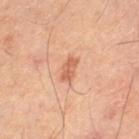Part of a total-body skin-imaging series; this lesion was reviewed on a skin check and was not flagged for biopsy. A male subject, aged approximately 65. From the left thigh. Captured under cross-polarized illumination. A 15 mm close-up extracted from a 3D total-body photography capture. The total-body-photography lesion software estimated a lesion area of about 4 mm², a shape eccentricity near 0.85, and two-axis asymmetry of about 0.2. The software also gave a lesion–skin lightness drop of about 10 and a normalized border contrast of about 6.5. The software also gave an automated nevus-likeness rating near 40 out of 100. The recorded lesion diameter is about 3 mm.